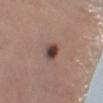Clinical impression:
This lesion was catalogued during total-body skin photography and was not selected for biopsy.
Clinical summary:
A 15 mm crop from a total-body photograph taken for skin-cancer surveillance. About 2 mm across. Automated image analysis of the tile measured an eccentricity of roughly 0.45 and two-axis asymmetry of about 0.2. It also reported an average lesion color of about L≈41 a*≈18 b*≈20 (CIELAB), roughly 15 lightness units darker than nearby skin, and a lesion-to-skin contrast of about 12 (normalized; higher = more distinct). Imaged with white-light lighting. A female patient aged 58–62. Located on the left lower leg.